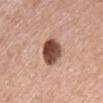Recorded during total-body skin imaging; not selected for excision or biopsy.
Captured under white-light illumination.
Located on the chest.
The lesion's longest dimension is about 3.5 mm.
A 15 mm close-up extracted from a 3D total-body photography capture.
A female subject aged approximately 50.